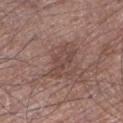Imaged during a routine full-body skin examination; the lesion was not biopsied and no histopathology is available. Located on the left lower leg. Captured under white-light illumination. Measured at roughly 4.5 mm in maximum diameter. The subject is a male in their mid-70s. An algorithmic analysis of the crop reported a footprint of about 6.5 mm², a shape eccentricity near 0.9, and a symmetry-axis asymmetry near 0.45. It also reported a lesion-detection confidence of about 95/100. This image is a 15 mm lesion crop taken from a total-body photograph.